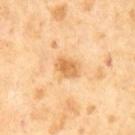biopsy status: catalogued during a skin exam; not biopsied
diameter: about 3 mm
subject: male, in their mid-60s
image source: ~15 mm crop, total-body skin-cancer survey
automated metrics: an area of roughly 6 mm², an eccentricity of roughly 0.65, and a shape-asymmetry score of about 0.15 (0 = symmetric); a lesion color around L≈67 a*≈22 b*≈44 in CIELAB, about 11 CIELAB-L* units darker than the surrounding skin, and a lesion-to-skin contrast of about 7 (normalized; higher = more distinct); a border-irregularity index near 1.5/10, a color-variation rating of about 3.5/10, and radial color variation of about 1; an automated nevus-likeness rating near 10 out of 100 and a detector confidence of about 100 out of 100 that the crop contains a lesion